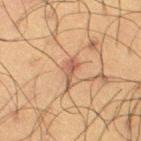This lesion was catalogued during total-body skin photography and was not selected for biopsy.
The subject is a male aged around 60.
On the right thigh.
Automated image analysis of the tile measured a footprint of about 4 mm², an eccentricity of roughly 0.9, and a shape-asymmetry score of about 0.45 (0 = symmetric).
Approximately 3.5 mm at its widest.
This is a cross-polarized tile.
Cropped from a whole-body photographic skin survey; the tile spans about 15 mm.